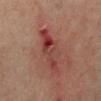This lesion was catalogued during total-body skin photography and was not selected for biopsy. The lesion is on the left lower leg. The lesion-visualizer software estimated a lesion color around L≈40 a*≈27 b*≈24 in CIELAB, roughly 9 lightness units darker than nearby skin, and a normalized lesion–skin contrast near 7.5. The analysis additionally found a border-irregularity index near 3.5/10, a within-lesion color-variation index near 10/10, and radial color variation of about 3. The lesion's longest dimension is about 6.5 mm. A roughly 15 mm field-of-view crop from a total-body skin photograph. A female subject approximately 65 years of age.